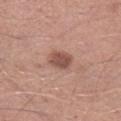The lesion was tiled from a total-body skin photograph and was not biopsied. The recorded lesion diameter is about 3 mm. This is a white-light tile. A roughly 15 mm field-of-view crop from a total-body skin photograph. The lesion is on the right lower leg. The patient is a male in their mid- to late 40s.